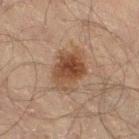<tbp_lesion>
  <biopsy_status>not biopsied; imaged during a skin examination</biopsy_status>
  <patient>
    <sex>male</sex>
    <age_approx>60</age_approx>
  </patient>
  <image>
    <source>total-body photography crop</source>
    <field_of_view_mm>15</field_of_view_mm>
  </image>
  <lesion_size>
    <long_diameter_mm_approx>5.0</long_diameter_mm_approx>
  </lesion_size>
  <site>right thigh</site>
</tbp_lesion>